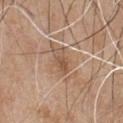A male patient aged 63 to 67.
On the chest.
The tile uses white-light illumination.
A 15 mm crop from a total-body photograph taken for skin-cancer surveillance.